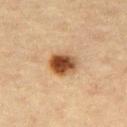Q: Is there a histopathology result?
A: total-body-photography surveillance lesion; no biopsy
Q: How large is the lesion?
A: ~3.5 mm (longest diameter)
Q: What is the anatomic site?
A: the right thigh
Q: What is the imaging modality?
A: 15 mm crop, total-body photography
Q: Automated lesion metrics?
A: an area of roughly 9 mm² and an eccentricity of roughly 0.6; a lesion color around L≈44 a*≈19 b*≈32 in CIELAB, a lesion–skin lightness drop of about 16, and a normalized lesion–skin contrast near 12
Q: What lighting was used for the tile?
A: cross-polarized
Q: Patient demographics?
A: female, in their mid- to late 60s